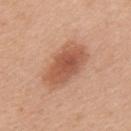biopsy status: catalogued during a skin exam; not biopsied | subject: female, aged around 40 | illumination: white-light illumination | body site: the upper back | imaging modality: 15 mm crop, total-body photography.